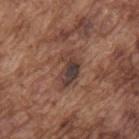  biopsy_status: not biopsied; imaged during a skin examination
  site: back
  patient:
    sex: male
    age_approx: 75
  image:
    source: total-body photography crop
    field_of_view_mm: 15